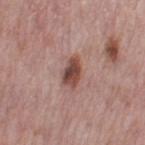| field | value |
|---|---|
| workup | catalogued during a skin exam; not biopsied |
| subject | female, aged approximately 50 |
| TBP lesion metrics | a shape eccentricity near 0.85; an average lesion color of about L≈46 a*≈22 b*≈24 (CIELAB) and a lesion-to-skin contrast of about 10 (normalized; higher = more distinct); a border-irregularity index near 3/10, internal color variation of about 4.5 on a 0–10 scale, and radial color variation of about 1.5 |
| lesion size | ~3.5 mm (longest diameter) |
| tile lighting | white-light illumination |
| site | the left thigh |
| image source | ~15 mm crop, total-body skin-cancer survey |